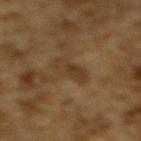The lesion was tiled from a total-body skin photograph and was not biopsied. An algorithmic analysis of the crop reported an eccentricity of roughly 0.75 and two-axis asymmetry of about 0.2. The software also gave an average lesion color of about L≈31 a*≈13 b*≈27 (CIELAB), a lesion–skin lightness drop of about 6, and a normalized lesion–skin contrast near 6. And it measured border irregularity of about 2.5 on a 0–10 scale, a within-lesion color-variation index near 3/10, and peripheral color asymmetry of about 1. The lesion is on the upper back. A 15 mm close-up extracted from a 3D total-body photography capture. A male patient aged 83 or over. The lesion's longest dimension is about 3.5 mm.